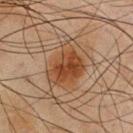{
  "biopsy_status": "not biopsied; imaged during a skin examination",
  "image": {
    "source": "total-body photography crop",
    "field_of_view_mm": 15
  },
  "site": "chest",
  "patient": {
    "sex": "male",
    "age_approx": 45
  },
  "lesion_size": {
    "long_diameter_mm_approx": 5.5
  },
  "automated_metrics": {
    "vs_skin_darker_L": 9.0,
    "lesion_detection_confidence_0_100": 100
  }
}